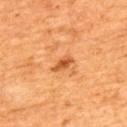No biopsy was performed on this lesion — it was imaged during a full skin examination and was not determined to be concerning. Measured at roughly 3 mm in maximum diameter. The patient is a male in their mid-60s. This image is a 15 mm lesion crop taken from a total-body photograph. The tile uses cross-polarized illumination. The lesion is located on the upper back.